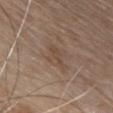biopsy status: catalogued during a skin exam; not biopsied | image source: ~15 mm crop, total-body skin-cancer survey | illumination: white-light illumination | site: the front of the torso | lesion size: ≈4 mm | patient: female, in their mid-60s.